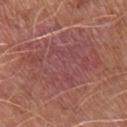Clinical impression: Captured during whole-body skin photography for melanoma surveillance; the lesion was not biopsied. Context: From the right lower leg. A close-up tile cropped from a whole-body skin photograph, about 15 mm across. The patient is a male in their mid- to late 60s.